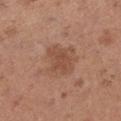notes — imaged on a skin check; not biopsied | tile lighting — white-light | imaging modality — ~15 mm crop, total-body skin-cancer survey | size — about 4.5 mm | patient — female, roughly 30 years of age | site — the right lower leg.